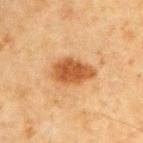Clinical impression: Part of a total-body skin-imaging series; this lesion was reviewed on a skin check and was not flagged for biopsy. Clinical summary: Approximately 5 mm at its widest. From the front of the torso. A male subject, aged around 65. The tile uses cross-polarized illumination. Cropped from a total-body skin-imaging series; the visible field is about 15 mm.